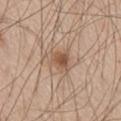Assessment:
No biopsy was performed on this lesion — it was imaged during a full skin examination and was not determined to be concerning.
Clinical summary:
The lesion is on the right thigh. A male patient, aged 58–62. A 15 mm close-up tile from a total-body photography series done for melanoma screening. This is a white-light tile. Approximately 2.5 mm at its widest. The total-body-photography lesion software estimated a lesion area of about 4.5 mm², an eccentricity of roughly 0.6, and a symmetry-axis asymmetry near 0.35. The software also gave a lesion–skin lightness drop of about 10 and a normalized lesion–skin contrast near 7.5. The analysis additionally found border irregularity of about 3.5 on a 0–10 scale, a within-lesion color-variation index near 3.5/10, and peripheral color asymmetry of about 1. The software also gave a classifier nevus-likeness of about 85/100 and a detector confidence of about 100 out of 100 that the crop contains a lesion.